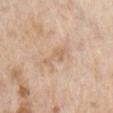biopsy status: catalogued during a skin exam; not biopsied
imaging modality: ~15 mm tile from a whole-body skin photo
TBP lesion metrics: a lesion color around L≈65 a*≈17 b*≈32 in CIELAB, a lesion–skin lightness drop of about 7, and a lesion-to-skin contrast of about 5 (normalized; higher = more distinct); a border-irregularity index near 4.5/10, a within-lesion color-variation index near 1/10, and a peripheral color-asymmetry measure near 0.5; a nevus-likeness score of about 0/100 and a lesion-detection confidence of about 100/100
site: the chest
subject: male, aged approximately 60
lesion diameter: ~4.5 mm (longest diameter)
illumination: white-light